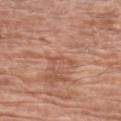workup: imaged on a skin check; not biopsied
lesion size: ~3.5 mm (longest diameter)
automated lesion analysis: an average lesion color of about L≈54 a*≈24 b*≈31 (CIELAB), about 7 CIELAB-L* units darker than the surrounding skin, and a lesion-to-skin contrast of about 5 (normalized; higher = more distinct); a within-lesion color-variation index near 0/10 and peripheral color asymmetry of about 0
image source: ~15 mm crop, total-body skin-cancer survey
illumination: white-light illumination
body site: the left forearm
subject: male, aged approximately 70